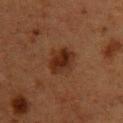Imaged during a routine full-body skin examination; the lesion was not biopsied and no histopathology is available. This image is a 15 mm lesion crop taken from a total-body photograph. Captured under cross-polarized illumination. Located on the upper back. The patient is a female approximately 40 years of age. Approximately 4 mm at its widest. The total-body-photography lesion software estimated a shape eccentricity near 0.65 and two-axis asymmetry of about 0.15. It also reported a lesion color around L≈24 a*≈19 b*≈25 in CIELAB. It also reported a border-irregularity index near 2/10, a color-variation rating of about 4.5/10, and radial color variation of about 1.5.